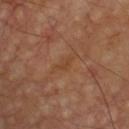This lesion was catalogued during total-body skin photography and was not selected for biopsy. The subject is a male aged 58–62. From the chest. A region of skin cropped from a whole-body photographic capture, roughly 15 mm wide.